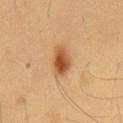Impression:
Recorded during total-body skin imaging; not selected for excision or biopsy.
Image and clinical context:
The recorded lesion diameter is about 3.5 mm. On the chest. A 15 mm crop from a total-body photograph taken for skin-cancer surveillance. Automated tile analysis of the lesion measured an area of roughly 7 mm². The analysis additionally found an average lesion color of about L≈52 a*≈22 b*≈39 (CIELAB), a lesion–skin lightness drop of about 14, and a normalized border contrast of about 9.5. The software also gave a nevus-likeness score of about 100/100. The subject is a male in their mid-50s. Imaged with cross-polarized lighting.